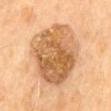notes = total-body-photography surveillance lesion; no biopsy | image = 15 mm crop, total-body photography | image-analysis metrics = peripheral color asymmetry of about 2.5; a nevus-likeness score of about 25/100 and a lesion-detection confidence of about 100/100 | lesion size = ≈8.5 mm | patient = male, roughly 70 years of age | location = the mid back | lighting = cross-polarized illumination.